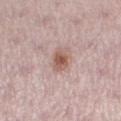image source: 15 mm crop, total-body photography | lesion diameter: about 3 mm | TBP lesion metrics: an automated nevus-likeness rating near 85 out of 100 and a detector confidence of about 100 out of 100 that the crop contains a lesion | lighting: white-light | location: the left lower leg | patient: female, aged around 30.